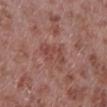Notes:
- biopsy status · catalogued during a skin exam; not biopsied
- imaging modality · ~15 mm crop, total-body skin-cancer survey
- location · the left lower leg
- subject · male, aged around 55